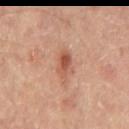This lesion was catalogued during total-body skin photography and was not selected for biopsy. A male patient, aged around 65. A 15 mm close-up tile from a total-body photography series done for melanoma screening. Imaged with cross-polarized lighting. The lesion is on the back. Measured at roughly 3.5 mm in maximum diameter.